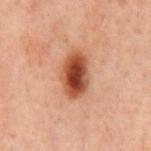workup = catalogued during a skin exam; not biopsied